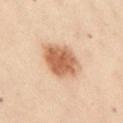Impression:
The lesion was photographed on a routine skin check and not biopsied; there is no pathology result.
Acquisition and patient details:
The lesion is located on the abdomen. The total-body-photography lesion software estimated a mean CIELAB color near L≈49 a*≈18 b*≈29, roughly 12 lightness units darker than nearby skin, and a lesion-to-skin contrast of about 9.5 (normalized; higher = more distinct). And it measured a nevus-likeness score of about 100/100 and a detector confidence of about 100 out of 100 that the crop contains a lesion. Captured under cross-polarized illumination. The subject is a female about 30 years old. A region of skin cropped from a whole-body photographic capture, roughly 15 mm wide.